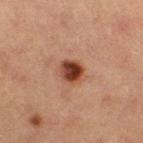follow-up=imaged on a skin check; not biopsied
patient=female, aged around 40
location=the leg
imaging modality=15 mm crop, total-body photography